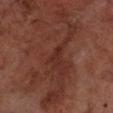Q: Was a biopsy performed?
A: catalogued during a skin exam; not biopsied
Q: What is the lesion's diameter?
A: ≈2.5 mm
Q: Automated lesion metrics?
A: a lesion area of about 3 mm² and a shape eccentricity near 0.85; border irregularity of about 4 on a 0–10 scale, internal color variation of about 0 on a 0–10 scale, and peripheral color asymmetry of about 0; an automated nevus-likeness rating near 0 out of 100 and a lesion-detection confidence of about 55/100
Q: What are the patient's age and sex?
A: male, aged around 70
Q: How was this image acquired?
A: total-body-photography crop, ~15 mm field of view
Q: Lesion location?
A: the right lower leg
Q: Illumination type?
A: cross-polarized